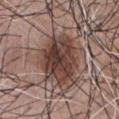Image and clinical context:
The recorded lesion diameter is about 7 mm. This is a white-light tile. A 15 mm close-up extracted from a 3D total-body photography capture. From the chest. The lesion-visualizer software estimated a mean CIELAB color near L≈40 a*≈18 b*≈22 and about 14 CIELAB-L* units darker than the surrounding skin. The patient is a male about 75 years old.